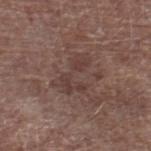{
  "biopsy_status": "not biopsied; imaged during a skin examination",
  "site": "right lower leg",
  "image": {
    "source": "total-body photography crop",
    "field_of_view_mm": 15
  },
  "automated_metrics": {
    "cielab_L": 39,
    "cielab_a": 18,
    "cielab_b": 21,
    "vs_skin_darker_L": 6.0,
    "vs_skin_contrast_norm": 5.5,
    "border_irregularity_0_10": 8.0,
    "color_variation_0_10": 2.5
  },
  "patient": {
    "sex": "male",
    "age_approx": 70
  },
  "lighting": "white-light",
  "lesion_size": {
    "long_diameter_mm_approx": 4.5
  }
}